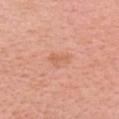<lesion>
  <biopsy_status>not biopsied; imaged during a skin examination</biopsy_status>
  <lighting>white-light</lighting>
  <lesion_size>
    <long_diameter_mm_approx>3.0</long_diameter_mm_approx>
  </lesion_size>
  <image>
    <source>total-body photography crop</source>
    <field_of_view_mm>15</field_of_view_mm>
  </image>
  <site>head or neck</site>
  <automated_metrics>
    <area_mm2_approx>4.0</area_mm2_approx>
    <eccentricity>0.8</eccentricity>
    <shape_asymmetry>0.4</shape_asymmetry>
    <nevus_likeness_0_100>5</nevus_likeness_0_100>
    <lesion_detection_confidence_0_100>100</lesion_detection_confidence_0_100>
  </automated_metrics>
  <patient>
    <sex>female</sex>
    <age_approx>60</age_approx>
  </patient>
</lesion>